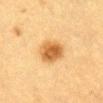Clinical impression: The lesion was tiled from a total-body skin photograph and was not biopsied. Acquisition and patient details: Measured at roughly 4 mm in maximum diameter. The subject is a female roughly 55 years of age. Automated image analysis of the tile measured an average lesion color of about L≈51 a*≈20 b*≈40 (CIELAB) and a normalized border contrast of about 9. The software also gave a lesion-detection confidence of about 100/100. On the abdomen. A 15 mm crop from a total-body photograph taken for skin-cancer surveillance.